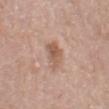Findings:
* follow-up: total-body-photography surveillance lesion; no biopsy
* anatomic site: the left upper arm
* TBP lesion metrics: a classifier nevus-likeness of about 40/100 and a lesion-detection confidence of about 100/100
* lesion size: about 3.5 mm
* illumination: white-light
* patient: male, in their mid-60s
* image: total-body-photography crop, ~15 mm field of view